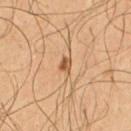Longest diameter approximately 2.5 mm. Located on the chest. The subject is a male aged 63–67. An algorithmic analysis of the crop reported an area of roughly 3 mm² and an outline eccentricity of about 0.7 (0 = round, 1 = elongated). It also reported a nevus-likeness score of about 85/100 and a detector confidence of about 100 out of 100 that the crop contains a lesion. This image is a 15 mm lesion crop taken from a total-body photograph.